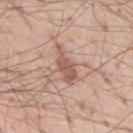Case summary:
- follow-up: catalogued during a skin exam; not biopsied
- image source: ~15 mm tile from a whole-body skin photo
- subject: male, in their mid- to late 50s
- anatomic site: the mid back